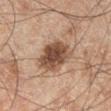The lesion was tiled from a total-body skin photograph and was not biopsied. A male subject, aged 43–47. Automated tile analysis of the lesion measured a footprint of about 14 mm² and an eccentricity of roughly 0.65. And it measured a classifier nevus-likeness of about 85/100 and a lesion-detection confidence of about 100/100. Imaged with cross-polarized lighting. The recorded lesion diameter is about 5 mm. Located on the left lower leg. Cropped from a whole-body photographic skin survey; the tile spans about 15 mm.